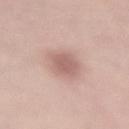Q: Was this lesion biopsied?
A: imaged on a skin check; not biopsied
Q: Automated lesion metrics?
A: a mean CIELAB color near L≈61 a*≈20 b*≈24 and a normalized border contrast of about 7
Q: How was this image acquired?
A: 15 mm crop, total-body photography
Q: Lesion location?
A: the lower back
Q: Who is the patient?
A: female, approximately 40 years of age
Q: What lighting was used for the tile?
A: white-light illumination